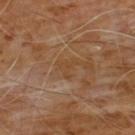  biopsy_status: not biopsied; imaged during a skin examination
  patient:
    sex: male
    age_approx: 60
  automated_metrics:
    area_mm2_approx: 3.0
    eccentricity: 0.6
    shape_asymmetry: 0.6
  lighting: cross-polarized
  image:
    source: total-body photography crop
    field_of_view_mm: 15
  lesion_size:
    long_diameter_mm_approx: 2.5
  site: chest